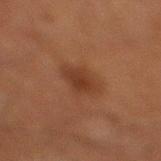Findings:
– notes: catalogued during a skin exam; not biopsied
– subject: male, roughly 65 years of age
– image: 15 mm crop, total-body photography
– anatomic site: the left lower leg